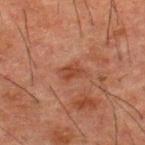Background:
A 15 mm close-up extracted from a 3D total-body photography capture. The lesion is on the upper back. The total-body-photography lesion software estimated an area of roughly 5 mm² and a shape-asymmetry score of about 0.45 (0 = symmetric). And it measured a lesion color around L≈35 a*≈21 b*≈26 in CIELAB, roughly 7 lightness units darker than nearby skin, and a normalized border contrast of about 6.5. The analysis additionally found a detector confidence of about 100 out of 100 that the crop contains a lesion. A male patient, aged 48–52. This is a cross-polarized tile.